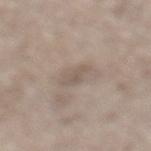No biopsy was performed on this lesion — it was imaged during a full skin examination and was not determined to be concerning.
A roughly 15 mm field-of-view crop from a total-body skin photograph.
Imaged with white-light lighting.
Longest diameter approximately 2.5 mm.
On the left lower leg.
A male subject in their 70s.